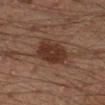Q: Was this lesion biopsied?
A: no biopsy performed (imaged during a skin exam)
Q: What lighting was used for the tile?
A: cross-polarized illumination
Q: What are the patient's age and sex?
A: male, about 45 years old
Q: What did automated image analysis measure?
A: an average lesion color of about L≈26 a*≈16 b*≈21 (CIELAB), roughly 8 lightness units darker than nearby skin, and a normalized border contrast of about 9; a border-irregularity index near 2.5/10, internal color variation of about 2.5 on a 0–10 scale, and peripheral color asymmetry of about 0.5; a nevus-likeness score of about 60/100 and a detector confidence of about 100 out of 100 that the crop contains a lesion
Q: Lesion location?
A: the right lower leg
Q: What kind of image is this?
A: ~15 mm tile from a whole-body skin photo